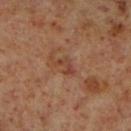Assessment:
No biopsy was performed on this lesion — it was imaged during a full skin examination and was not determined to be concerning.
Background:
A close-up tile cropped from a whole-body skin photograph, about 15 mm across. The total-body-photography lesion software estimated a shape eccentricity near 0.8 and a shape-asymmetry score of about 0.55 (0 = symmetric). It also reported border irregularity of about 6 on a 0–10 scale, internal color variation of about 0 on a 0–10 scale, and a peripheral color-asymmetry measure near 0. On the right lower leg. The recorded lesion diameter is about 2.5 mm. The patient is a male aged 58 to 62.